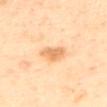Q: Is there a histopathology result?
A: no biopsy performed (imaged during a skin exam)
Q: Who is the patient?
A: male, about 65 years old
Q: What kind of image is this?
A: total-body-photography crop, ~15 mm field of view
Q: Lesion location?
A: the mid back